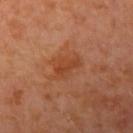The lesion was photographed on a routine skin check and not biopsied; there is no pathology result. The lesion is located on the right upper arm. The patient is a female approximately 50 years of age. A roughly 15 mm field-of-view crop from a total-body skin photograph.